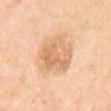Impression:
This lesion was catalogued during total-body skin photography and was not selected for biopsy.
Context:
A 15 mm close-up extracted from a 3D total-body photography capture. On the abdomen. The subject is a female roughly 55 years of age. The total-body-photography lesion software estimated an area of roughly 18 mm² and an outline eccentricity of about 0.35 (0 = round, 1 = elongated). And it measured a border-irregularity index near 2.5/10, a within-lesion color-variation index near 5/10, and a peripheral color-asymmetry measure near 2. This is a cross-polarized tile.